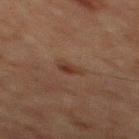Case summary:
– size · about 2.5 mm
– subject · male, roughly 65 years of age
– imaging modality · ~15 mm tile from a whole-body skin photo
– lighting · cross-polarized illumination
– location · the chest